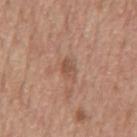{
  "biopsy_status": "not biopsied; imaged during a skin examination",
  "site": "chest",
  "patient": {
    "sex": "male",
    "age_approx": 70
  },
  "image": {
    "source": "total-body photography crop",
    "field_of_view_mm": 15
  },
  "automated_metrics": {
    "area_mm2_approx": 3.5,
    "shape_asymmetry": 0.2,
    "cielab_L": 52,
    "cielab_a": 20,
    "cielab_b": 29,
    "vs_skin_darker_L": 8.0,
    "vs_skin_contrast_norm": 6.0,
    "border_irregularity_0_10": 2.0,
    "color_variation_0_10": 2.0
  }
}